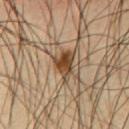Part of a total-body skin-imaging series; this lesion was reviewed on a skin check and was not flagged for biopsy.
A close-up tile cropped from a whole-body skin photograph, about 15 mm across.
A male subject aged approximately 40.
The tile uses cross-polarized illumination.
The lesion-visualizer software estimated an eccentricity of roughly 0.75 and a shape-asymmetry score of about 0.4 (0 = symmetric). The software also gave a mean CIELAB color near L≈45 a*≈17 b*≈32 and a normalized lesion–skin contrast near 11. It also reported a border-irregularity rating of about 4.5/10 and a color-variation rating of about 6.5/10. And it measured an automated nevus-likeness rating near 90 out of 100 and a lesion-detection confidence of about 100/100.
Located on the chest.
Measured at roughly 4 mm in maximum diameter.